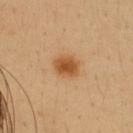Notes:
• biopsy status — imaged on a skin check; not biopsied
• patient — female, aged 33 to 37
• TBP lesion metrics — peripheral color asymmetry of about 1; a nevus-likeness score of about 100/100 and a lesion-detection confidence of about 100/100
• tile lighting — cross-polarized illumination
• imaging modality — total-body-photography crop, ~15 mm field of view
• location — the upper back